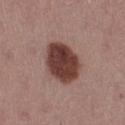| feature | finding |
|---|---|
| lighting | white-light |
| imaging modality | ~15 mm tile from a whole-body skin photo |
| subject | female, about 55 years old |
| body site | the left thigh |